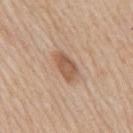Impression:
The lesion was tiled from a total-body skin photograph and was not biopsied.
Clinical summary:
Located on the back. This image is a 15 mm lesion crop taken from a total-body photograph. The subject is a male about 60 years old. The tile uses white-light illumination. The lesion's longest dimension is about 4 mm.